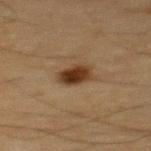notes = catalogued during a skin exam; not biopsied | image = ~15 mm crop, total-body skin-cancer survey | site = the upper back | automated metrics = an average lesion color of about L≈29 a*≈16 b*≈27 (CIELAB), a lesion–skin lightness drop of about 13, and a normalized lesion–skin contrast near 12; a lesion-detection confidence of about 100/100 | lesion size = ≈3 mm | tile lighting = cross-polarized illumination | subject = male, aged 63–67.